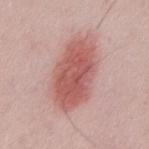{
  "biopsy_status": "not biopsied; imaged during a skin examination",
  "lighting": "white-light",
  "lesion_size": {
    "long_diameter_mm_approx": 8.0
  },
  "image": {
    "source": "total-body photography crop",
    "field_of_view_mm": 15
  },
  "site": "abdomen",
  "patient": {
    "sex": "male",
    "age_approx": 50
  }
}